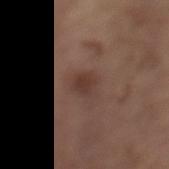Q: How large is the lesion?
A: ≈2.5 mm
Q: Illumination type?
A: white-light illumination
Q: How was this image acquired?
A: ~15 mm tile from a whole-body skin photo
Q: Who is the patient?
A: female, aged 63–67
Q: Lesion location?
A: the left lower leg